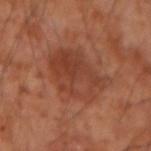This lesion was catalogued during total-body skin photography and was not selected for biopsy. The tile uses cross-polarized illumination. Longest diameter approximately 6 mm. From the arm. A male subject approximately 55 years of age. A 15 mm crop from a total-body photograph taken for skin-cancer surveillance. The lesion-visualizer software estimated a lesion color around L≈42 a*≈25 b*≈31 in CIELAB, about 7 CIELAB-L* units darker than the surrounding skin, and a normalized border contrast of about 6.